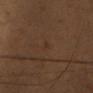{"biopsy_status": "not biopsied; imaged during a skin examination", "site": "chest", "lesion_size": {"long_diameter_mm_approx": 1.0}, "patient": {"sex": "female", "age_approx": 35}, "image": {"source": "total-body photography crop", "field_of_view_mm": 15}}